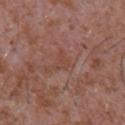workup=no biopsy performed (imaged during a skin exam) | illumination=white-light illumination | patient=male, about 45 years old | body site=the chest | lesion size=~2.5 mm (longest diameter) | acquisition=~15 mm tile from a whole-body skin photo | image-analysis metrics=a lesion area of about 3 mm², an eccentricity of roughly 0.65, and a symmetry-axis asymmetry near 0.65; a border-irregularity index near 6/10, a within-lesion color-variation index near 1/10, and peripheral color asymmetry of about 0.5.